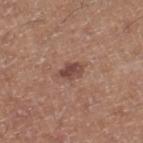The lesion was tiled from a total-body skin photograph and was not biopsied.
The patient is a male aged 48 to 52.
A region of skin cropped from a whole-body photographic capture, roughly 15 mm wide.
Located on the right lower leg.
The lesion-visualizer software estimated a mean CIELAB color near L≈46 a*≈20 b*≈24, a lesion–skin lightness drop of about 10, and a lesion-to-skin contrast of about 8 (normalized; higher = more distinct). The analysis additionally found a lesion-detection confidence of about 100/100.
Approximately 3 mm at its widest.